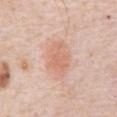Notes:
* follow-up — no biopsy performed (imaged during a skin exam)
* patient — male, approximately 80 years of age
* tile lighting — white-light
* imaging modality — ~15 mm crop, total-body skin-cancer survey
* lesion diameter — about 5 mm
* site — the abdomen